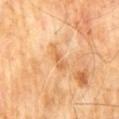biopsy_status: not biopsied; imaged during a skin examination
site: abdomen
patient:
  sex: male
  age_approx: 60
image:
  source: total-body photography crop
  field_of_view_mm: 15